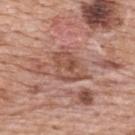{
  "image": {
    "source": "total-body photography crop",
    "field_of_view_mm": 15
  },
  "lighting": "white-light",
  "patient": {
    "sex": "female",
    "age_approx": 60
  },
  "site": "upper back"
}